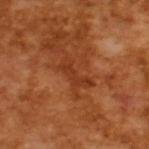The lesion was photographed on a routine skin check and not biopsied; there is no pathology result.
An algorithmic analysis of the crop reported a footprint of about 5.5 mm² and a symmetry-axis asymmetry near 0.45. The software also gave a lesion color around L≈36 a*≈28 b*≈37 in CIELAB, roughly 7 lightness units darker than nearby skin, and a normalized border contrast of about 6. The software also gave a border-irregularity index near 6/10, internal color variation of about 1 on a 0–10 scale, and radial color variation of about 0. It also reported a nevus-likeness score of about 0/100 and lesion-presence confidence of about 95/100.
The patient is a male aged 63–67.
Longest diameter approximately 4 mm.
Cropped from a whole-body photographic skin survey; the tile spans about 15 mm.